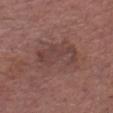Q: Was a biopsy performed?
A: catalogued during a skin exam; not biopsied
Q: How was this image acquired?
A: ~15 mm crop, total-body skin-cancer survey
Q: Lesion location?
A: the chest
Q: What did automated image analysis measure?
A: a lesion color around L≈42 a*≈19 b*≈21 in CIELAB, roughly 6 lightness units darker than nearby skin, and a lesion-to-skin contrast of about 5 (normalized; higher = more distinct)
Q: What is the lesion's diameter?
A: ≈6 mm
Q: Patient demographics?
A: male, aged around 80
Q: Illumination type?
A: white-light